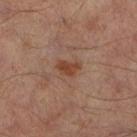| key | value |
|---|---|
| biopsy status | imaged on a skin check; not biopsied |
| patient | male, aged around 65 |
| site | the right lower leg |
| acquisition | ~15 mm tile from a whole-body skin photo |
| illumination | cross-polarized illumination |